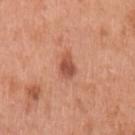Imaged during a routine full-body skin examination; the lesion was not biopsied and no histopathology is available. From the arm. The recorded lesion diameter is about 2.5 mm. The subject is a female in their mid-60s. A close-up tile cropped from a whole-body skin photograph, about 15 mm across. Captured under white-light illumination. The lesion-visualizer software estimated a mean CIELAB color near L≈52 a*≈28 b*≈32. It also reported a border-irregularity rating of about 2/10 and radial color variation of about 1.